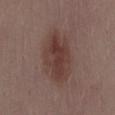– notes · total-body-photography surveillance lesion; no biopsy
– lighting · white-light
– location · the front of the torso
– automated metrics · an automated nevus-likeness rating near 80 out of 100 and a lesion-detection confidence of about 100/100
– lesion size · about 7 mm
– imaging modality · total-body-photography crop, ~15 mm field of view
– patient · female, aged approximately 30